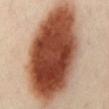acquisition = total-body-photography crop, ~15 mm field of view
tile lighting = cross-polarized illumination
TBP lesion metrics = an area of roughly 80 mm², a shape eccentricity near 0.9, and two-axis asymmetry of about 0.1; an average lesion color of about L≈48 a*≈24 b*≈31 (CIELAB), a lesion–skin lightness drop of about 23, and a normalized border contrast of about 15.5; a border-irregularity index near 2/10 and internal color variation of about 10 on a 0–10 scale
anatomic site = the mid back
size = about 16 mm
subject = male, aged approximately 50